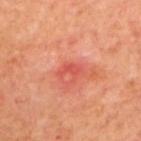The lesion was tiled from a total-body skin photograph and was not biopsied.
The subject is a male aged 68 to 72.
The lesion is located on the upper back.
The recorded lesion diameter is about 2.5 mm.
Automated image analysis of the tile measured an area of roughly 2.5 mm² and a shape-asymmetry score of about 0.55 (0 = symmetric).
A 15 mm close-up tile from a total-body photography series done for melanoma screening.